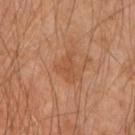Findings:
* workup — imaged on a skin check; not biopsied
* size — about 3.5 mm
* site — the left forearm
* acquisition — ~15 mm tile from a whole-body skin photo
* subject — male, in their mid-40s